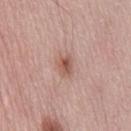Assessment: The lesion was tiled from a total-body skin photograph and was not biopsied. Context: A male subject, approximately 65 years of age. A 15 mm close-up tile from a total-body photography series done for melanoma screening. Approximately 2.5 mm at its widest. Automated tile analysis of the lesion measured an average lesion color of about L≈56 a*≈21 b*≈27 (CIELAB) and a lesion–skin lightness drop of about 10. And it measured a detector confidence of about 100 out of 100 that the crop contains a lesion. The lesion is on the mid back. Imaged with white-light lighting.